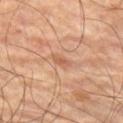The lesion was photographed on a routine skin check and not biopsied; there is no pathology result.
A 15 mm close-up extracted from a 3D total-body photography capture.
Located on the left thigh.
About 2.5 mm across.
This is a cross-polarized tile.
A male patient in their mid-60s.
Automated tile analysis of the lesion measured a mean CIELAB color near L≈56 a*≈22 b*≈32, a lesion–skin lightness drop of about 8, and a normalized border contrast of about 5.5. The analysis additionally found radial color variation of about 0.